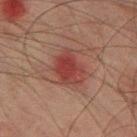Q: Was this lesion biopsied?
A: imaged on a skin check; not biopsied
Q: How was this image acquired?
A: ~15 mm tile from a whole-body skin photo
Q: Automated lesion metrics?
A: a footprint of about 10 mm², an outline eccentricity of about 0.7 (0 = round, 1 = elongated), and two-axis asymmetry of about 0.25; a border-irregularity rating of about 2.5/10, a within-lesion color-variation index near 3.5/10, and a peripheral color-asymmetry measure near 1
Q: Lesion location?
A: the left thigh
Q: What are the patient's age and sex?
A: male, approximately 75 years of age